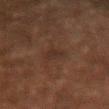workup: total-body-photography surveillance lesion; no biopsy.